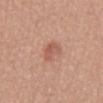The tile uses white-light illumination.
From the abdomen.
Automated tile analysis of the lesion measured a lesion area of about 4.5 mm² and a shape-asymmetry score of about 0.35 (0 = symmetric). It also reported a lesion color around L≈56 a*≈24 b*≈29 in CIELAB, roughly 8 lightness units darker than nearby skin, and a normalized border contrast of about 6. The software also gave a border-irregularity rating of about 3/10, a color-variation rating of about 2.5/10, and peripheral color asymmetry of about 1. The software also gave an automated nevus-likeness rating near 75 out of 100 and a detector confidence of about 100 out of 100 that the crop contains a lesion.
The patient is a female in their mid-50s.
The lesion's longest dimension is about 2.5 mm.
Cropped from a total-body skin-imaging series; the visible field is about 15 mm.